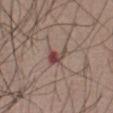Q: Was this lesion biopsied?
A: imaged on a skin check; not biopsied
Q: What did automated image analysis measure?
A: an area of roughly 4.5 mm² and two-axis asymmetry of about 0.4; a classifier nevus-likeness of about 0/100
Q: What is the anatomic site?
A: the abdomen
Q: What kind of image is this?
A: 15 mm crop, total-body photography
Q: What lighting was used for the tile?
A: white-light
Q: Patient demographics?
A: male, aged 53–57
Q: Lesion size?
A: ≈3 mm